This is a white-light tile; the lesion is located on the back; this image is a 15 mm lesion crop taken from a total-body photograph; a female subject aged 63 to 67 — 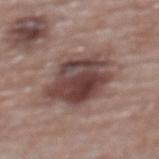• histopathologic diagnosis: a dysplastic (Clark) nevus